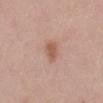| key | value |
|---|---|
| biopsy status | no biopsy performed (imaged during a skin exam) |
| body site | the abdomen |
| diameter | ~2.5 mm (longest diameter) |
| patient | female, about 50 years old |
| illumination | white-light |
| image source | ~15 mm crop, total-body skin-cancer survey |